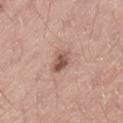Findings:
• follow-up — no biopsy performed (imaged during a skin exam)
• subject — male, aged approximately 20
• acquisition — ~15 mm tile from a whole-body skin photo
• body site — the leg